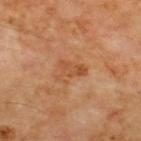Notes:
- workup: catalogued during a skin exam; not biopsied
- lighting: cross-polarized
- subject: male, aged around 70
- TBP lesion metrics: a lesion area of about 5.5 mm², an outline eccentricity of about 0.8 (0 = round, 1 = elongated), and a shape-asymmetry score of about 0.55 (0 = symmetric); a mean CIELAB color near L≈50 a*≈25 b*≈37; a classifier nevus-likeness of about 0/100 and a lesion-detection confidence of about 100/100
- anatomic site: the upper back
- diameter: about 3.5 mm
- acquisition: ~15 mm crop, total-body skin-cancer survey